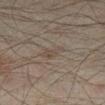{
  "biopsy_status": "not biopsied; imaged during a skin examination",
  "image": {
    "source": "total-body photography crop",
    "field_of_view_mm": 15
  },
  "patient": {
    "sex": "male",
    "age_approx": 45
  },
  "site": "right lower leg"
}